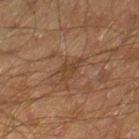notes=imaged on a skin check; not biopsied | subject=male, roughly 45 years of age | body site=the right lower leg | illumination=cross-polarized illumination | lesion diameter=about 3 mm | automated lesion analysis=a footprint of about 3.5 mm², an eccentricity of roughly 0.85, and two-axis asymmetry of about 0.5; an average lesion color of about L≈33 a*≈16 b*≈26 (CIELAB), a lesion–skin lightness drop of about 6, and a normalized border contrast of about 5.5 | image=total-body-photography crop, ~15 mm field of view.